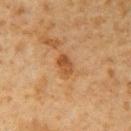No biopsy was performed on this lesion — it was imaged during a full skin examination and was not determined to be concerning. Imaged with cross-polarized lighting. A male subject roughly 60 years of age. Automated tile analysis of the lesion measured a lesion area of about 4.5 mm² and a symmetry-axis asymmetry near 0.25. The analysis additionally found an average lesion color of about L≈43 a*≈20 b*≈34 (CIELAB) and about 9 CIELAB-L* units darker than the surrounding skin. The analysis additionally found a classifier nevus-likeness of about 0/100. The lesion's longest dimension is about 3 mm. A 15 mm close-up extracted from a 3D total-body photography capture. Located on the right upper arm.